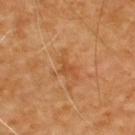Assessment: Part of a total-body skin-imaging series; this lesion was reviewed on a skin check and was not flagged for biopsy. Context: A male patient, approximately 60 years of age. Captured under cross-polarized illumination. The total-body-photography lesion software estimated a lesion color around L≈48 a*≈24 b*≈39 in CIELAB, about 7 CIELAB-L* units darker than the surrounding skin, and a lesion-to-skin contrast of about 5.5 (normalized; higher = more distinct). The software also gave a border-irregularity rating of about 7/10 and internal color variation of about 1 on a 0–10 scale. The analysis additionally found a lesion-detection confidence of about 100/100. Longest diameter approximately 3.5 mm. The lesion is located on the upper back. Cropped from a total-body skin-imaging series; the visible field is about 15 mm.